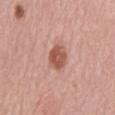notes: imaged on a skin check; not biopsied
patient: female, in their mid- to late 60s
image source: total-body-photography crop, ~15 mm field of view
location: the front of the torso